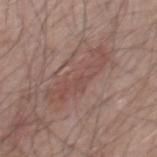Impression:
Captured during whole-body skin photography for melanoma surveillance; the lesion was not biopsied.
Context:
A 15 mm crop from a total-body photograph taken for skin-cancer surveillance. The subject is a male in their 70s. The lesion is located on the chest. Imaged with white-light lighting. The lesion's longest dimension is about 7 mm.